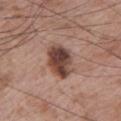Q: Was this lesion biopsied?
A: catalogued during a skin exam; not biopsied
Q: Illumination type?
A: white-light illumination
Q: How large is the lesion?
A: about 4.5 mm
Q: What is the anatomic site?
A: the left upper arm
Q: How was this image acquired?
A: total-body-photography crop, ~15 mm field of view
Q: What are the patient's age and sex?
A: male, in their mid-60s